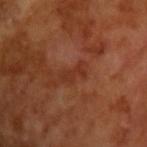Imaged during a routine full-body skin examination; the lesion was not biopsied and no histopathology is available.
This image is a 15 mm lesion crop taken from a total-body photograph.
A male patient about 65 years old.